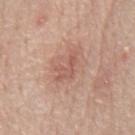biopsy status — total-body-photography surveillance lesion; no biopsy | automated lesion analysis — an automated nevus-likeness rating near 0 out of 100 and a detector confidence of about 100 out of 100 that the crop contains a lesion | patient — male, aged around 70 | image — total-body-photography crop, ~15 mm field of view | tile lighting — white-light | lesion diameter — ~4 mm (longest diameter) | body site — the front of the torso.